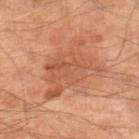Clinical impression:
The lesion was tiled from a total-body skin photograph and was not biopsied.
Background:
Measured at roughly 7.5 mm in maximum diameter. The lesion-visualizer software estimated a shape eccentricity near 0.75 and a shape-asymmetry score of about 0.4 (0 = symmetric). The analysis additionally found a lesion color around L≈54 a*≈25 b*≈35 in CIELAB, a lesion–skin lightness drop of about 8, and a lesion-to-skin contrast of about 5.5 (normalized; higher = more distinct). The software also gave a border-irregularity index near 6/10, a within-lesion color-variation index near 4/10, and a peripheral color-asymmetry measure near 1.5. And it measured an automated nevus-likeness rating near 0 out of 100. A 15 mm crop from a total-body photograph taken for skin-cancer surveillance. A male subject, in their mid-60s. The lesion is on the right lower leg.